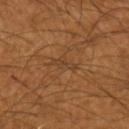The lesion was tiled from a total-body skin photograph and was not biopsied.
Captured under cross-polarized illumination.
A 15 mm crop from a total-body photograph taken for skin-cancer surveillance.
The lesion is on the left forearm.
Approximately 3 mm at its widest.
An algorithmic analysis of the crop reported a footprint of about 2.5 mm², a shape eccentricity near 0.95, and a shape-asymmetry score of about 0.45 (0 = symmetric). The analysis additionally found an average lesion color of about L≈38 a*≈19 b*≈32 (CIELAB) and a lesion-to-skin contrast of about 5.5 (normalized; higher = more distinct).
A male patient, aged around 55.